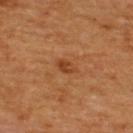| feature | finding |
|---|---|
| workup | total-body-photography surveillance lesion; no biopsy |
| subject | male, in their mid-60s |
| imaging modality | 15 mm crop, total-body photography |
| site | the upper back |
| lesion size | about 3 mm |